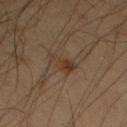Assessment:
The lesion was photographed on a routine skin check and not biopsied; there is no pathology result.
Background:
From the arm. A lesion tile, about 15 mm wide, cut from a 3D total-body photograph. Captured under cross-polarized illumination. A male patient, aged 58 to 62.